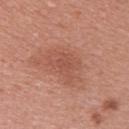Clinical impression:
This lesion was catalogued during total-body skin photography and was not selected for biopsy.
Clinical summary:
A 15 mm close-up extracted from a 3D total-body photography capture. From the back. A female subject aged 38 to 42. This is a white-light tile. An algorithmic analysis of the crop reported a footprint of about 9.5 mm² and a symmetry-axis asymmetry near 0.3. It also reported an average lesion color of about L≈53 a*≈25 b*≈29 (CIELAB) and roughly 7 lightness units darker than nearby skin. It also reported a border-irregularity index near 4/10, internal color variation of about 2 on a 0–10 scale, and peripheral color asymmetry of about 0.5.